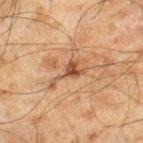- follow-up · no biopsy performed (imaged during a skin exam)
- imaging modality · total-body-photography crop, ~15 mm field of view
- patient · male, aged 43 to 47
- anatomic site · the right lower leg
- automated lesion analysis · an area of roughly 5.5 mm², an eccentricity of roughly 0.9, and two-axis asymmetry of about 0.6; a mean CIELAB color near L≈43 a*≈18 b*≈30, about 10 CIELAB-L* units darker than the surrounding skin, and a lesion-to-skin contrast of about 7.5 (normalized; higher = more distinct); a border-irregularity index near 8/10, a within-lesion color-variation index near 5/10, and peripheral color asymmetry of about 1.5; a nevus-likeness score of about 65/100 and lesion-presence confidence of about 100/100
- tile lighting · cross-polarized illumination